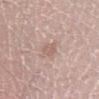{
  "image": {
    "source": "total-body photography crop",
    "field_of_view_mm": 15
  },
  "patient": {
    "sex": "male",
    "age_approx": 55
  },
  "lighting": "white-light",
  "site": "left lower leg"
}